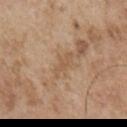biopsy status = total-body-photography surveillance lesion; no biopsy | body site = the right upper arm | subject = male, aged 53–57 | acquisition = total-body-photography crop, ~15 mm field of view | lesion diameter = ≈3.5 mm.